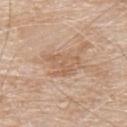notes=total-body-photography surveillance lesion; no biopsy
lesion diameter=about 4.5 mm
lighting=white-light illumination
subject=male, aged around 80
image=~15 mm crop, total-body skin-cancer survey
anatomic site=the back